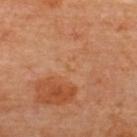Background:
A lesion tile, about 15 mm wide, cut from a 3D total-body photograph. The recorded lesion diameter is about 1 mm. This is a cross-polarized tile. The lesion is located on the upper back. The total-body-photography lesion software estimated a lesion color around L≈54 a*≈22 b*≈37 in CIELAB, roughly 3 lightness units darker than nearby skin, and a normalized lesion–skin contrast near 3. And it measured border irregularity of about 2.5 on a 0–10 scale, internal color variation of about 0 on a 0–10 scale, and radial color variation of about 0. And it measured a classifier nevus-likeness of about 0/100 and lesion-presence confidence of about 95/100. A female patient approximately 65 years of age.